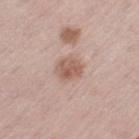Notes:
* follow-up: no biopsy performed (imaged during a skin exam)
* subject: female, aged 63–67
* anatomic site: the leg
* lesion diameter: ≈3 mm
* lighting: white-light illumination
* image source: ~15 mm crop, total-body skin-cancer survey